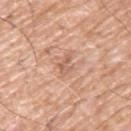Impression: Recorded during total-body skin imaging; not selected for excision or biopsy. Image and clinical context: This is a white-light tile. The patient is a male aged 58–62. From the left upper arm. Cropped from a total-body skin-imaging series; the visible field is about 15 mm.